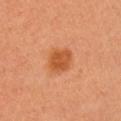workup — total-body-photography surveillance lesion; no biopsy
diameter — ~3 mm (longest diameter)
acquisition — total-body-photography crop, ~15 mm field of view
image-analysis metrics — a mean CIELAB color near L≈54 a*≈29 b*≈42, a lesion–skin lightness drop of about 10, and a normalized lesion–skin contrast near 8; a classifier nevus-likeness of about 95/100 and a lesion-detection confidence of about 100/100
patient — female, about 50 years old